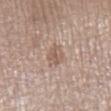Recorded during total-body skin imaging; not selected for excision or biopsy. The lesion-visualizer software estimated an area of roughly 4 mm², an eccentricity of roughly 0.6, and a symmetry-axis asymmetry near 0.25. The analysis additionally found a classifier nevus-likeness of about 0/100 and a detector confidence of about 100 out of 100 that the crop contains a lesion. A close-up tile cropped from a whole-body skin photograph, about 15 mm across. This is a white-light tile. Located on the right lower leg. A male patient in their mid- to late 70s. Approximately 2.5 mm at its widest.